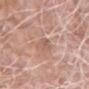<case>
<biopsy_status>not biopsied; imaged during a skin examination</biopsy_status>
<lesion_size>
  <long_diameter_mm_approx>3.0</long_diameter_mm_approx>
</lesion_size>
<image>
  <source>total-body photography crop</source>
  <field_of_view_mm>15</field_of_view_mm>
</image>
<lighting>white-light</lighting>
<site>left upper arm</site>
<patient>
  <sex>male</sex>
  <age_approx>75</age_approx>
</patient>
<automated_metrics>
  <area_mm2_approx>4.0</area_mm2_approx>
  <eccentricity>0.8</eccentricity>
  <shape_asymmetry>0.3</shape_asymmetry>
  <cielab_L>58</cielab_L>
  <cielab_a>21</cielab_a>
  <cielab_b>26</cielab_b>
  <vs_skin_darker_L>7.0</vs_skin_darker_L>
  <vs_skin_contrast_norm>5.0</vs_skin_contrast_norm>
  <border_irregularity_0_10>2.5</border_irregularity_0_10>
  <color_variation_0_10>2.0</color_variation_0_10>
  <peripheral_color_asymmetry>1.0</peripheral_color_asymmetry>
  <lesion_detection_confidence_0_100>85</lesion_detection_confidence_0_100>
</automated_metrics>
</case>